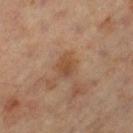<lesion>
<biopsy_status>not biopsied; imaged during a skin examination</biopsy_status>
<patient>
  <sex>male</sex>
  <age_approx>60</age_approx>
</patient>
<lesion_size>
  <long_diameter_mm_approx>3.0</long_diameter_mm_approx>
</lesion_size>
<lighting>cross-polarized</lighting>
<image>
  <source>total-body photography crop</source>
  <field_of_view_mm>15</field_of_view_mm>
</image>
<site>left thigh</site>
</lesion>